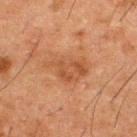Q: Was this lesion biopsied?
A: no biopsy performed (imaged during a skin exam)
Q: Patient demographics?
A: male, approximately 50 years of age
Q: Illumination type?
A: cross-polarized illumination
Q: What kind of image is this?
A: total-body-photography crop, ~15 mm field of view
Q: What is the anatomic site?
A: the upper back
Q: How large is the lesion?
A: about 5.5 mm
Q: What did automated image analysis measure?
A: an area of roughly 12 mm² and a symmetry-axis asymmetry near 0.3